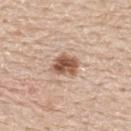Q: Is there a histopathology result?
A: no biopsy performed (imaged during a skin exam)
Q: What is the imaging modality?
A: ~15 mm crop, total-body skin-cancer survey
Q: Patient demographics?
A: male, approximately 60 years of age
Q: What is the anatomic site?
A: the upper back
Q: Automated lesion metrics?
A: a lesion area of about 6.5 mm², a shape eccentricity near 0.7, and a shape-asymmetry score of about 0.25 (0 = symmetric); an average lesion color of about L≈54 a*≈21 b*≈30 (CIELAB)